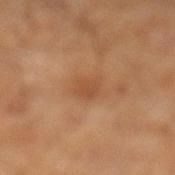Part of a total-body skin-imaging series; this lesion was reviewed on a skin check and was not flagged for biopsy.